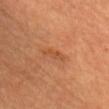Notes:
• follow-up · imaged on a skin check; not biopsied
• image · ~15 mm crop, total-body skin-cancer survey
• lesion diameter · ~3.5 mm (longest diameter)
• patient · female, aged 58 to 62
• tile lighting · cross-polarized
• site · the head or neck